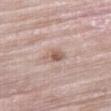| key | value |
|---|---|
| tile lighting | white-light |
| site | the right thigh |
| imaging modality | total-body-photography crop, ~15 mm field of view |
| lesion size | ~2.5 mm (longest diameter) |
| subject | male, roughly 80 years of age |
| image-analysis metrics | an average lesion color of about L≈58 a*≈18 b*≈25 (CIELAB), a lesion–skin lightness drop of about 10, and a normalized lesion–skin contrast near 7; a border-irregularity rating of about 3/10, internal color variation of about 4.5 on a 0–10 scale, and a peripheral color-asymmetry measure near 1.5; a classifier nevus-likeness of about 10/100 and a detector confidence of about 100 out of 100 that the crop contains a lesion |